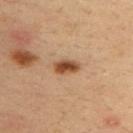The lesion was tiled from a total-body skin photograph and was not biopsied.
A lesion tile, about 15 mm wide, cut from a 3D total-body photograph.
About 3 mm across.
The subject is a male approximately 35 years of age.
The tile uses cross-polarized illumination.
The total-body-photography lesion software estimated a footprint of about 4.5 mm², a shape eccentricity near 0.8, and a symmetry-axis asymmetry near 0.2. The analysis additionally found a lesion color around L≈44 a*≈20 b*≈32 in CIELAB, about 14 CIELAB-L* units darker than the surrounding skin, and a normalized lesion–skin contrast near 10.5. It also reported border irregularity of about 2 on a 0–10 scale, a within-lesion color-variation index near 3.5/10, and radial color variation of about 1. The analysis additionally found a lesion-detection confidence of about 100/100.
The lesion is located on the upper back.